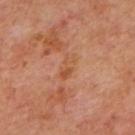lesion diameter — ≈3 mm
illumination — cross-polarized illumination
anatomic site — the upper back
acquisition — total-body-photography crop, ~15 mm field of view
patient — aged 63–67
TBP lesion metrics — an area of roughly 4 mm², an outline eccentricity of about 0.9 (0 = round, 1 = elongated), and a symmetry-axis asymmetry near 0.35; an average lesion color of about L≈52 a*≈25 b*≈36 (CIELAB) and a lesion-to-skin contrast of about 6 (normalized; higher = more distinct); a classifier nevus-likeness of about 0/100 and lesion-presence confidence of about 100/100